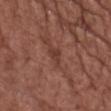Findings:
- biopsy status · imaged on a skin check; not biopsied
- site · the chest
- subject · male, in their mid- to late 70s
- acquisition · ~15 mm tile from a whole-body skin photo
- automated metrics · a footprint of about 3.5 mm² and an outline eccentricity of about 0.85 (0 = round, 1 = elongated); a mean CIELAB color near L≈38 a*≈23 b*≈25, roughly 7 lightness units darker than nearby skin, and a normalized border contrast of about 6.5; a border-irregularity rating of about 4.5/10, a within-lesion color-variation index near 1/10, and a peripheral color-asymmetry measure near 0.5
- tile lighting · white-light